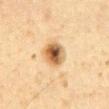This lesion was catalogued during total-body skin photography and was not selected for biopsy.
On the abdomen.
A male patient, roughly 60 years of age.
A region of skin cropped from a whole-body photographic capture, roughly 15 mm wide.
This is a cross-polarized tile.